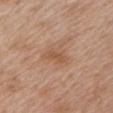Q: Patient demographics?
A: male, roughly 65 years of age
Q: What is the anatomic site?
A: the left upper arm
Q: What is the lesion's diameter?
A: ~3 mm (longest diameter)
Q: How was this image acquired?
A: ~15 mm crop, total-body skin-cancer survey